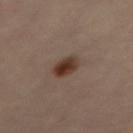Part of a total-body skin-imaging series; this lesion was reviewed on a skin check and was not flagged for biopsy.
From the abdomen.
A close-up tile cropped from a whole-body skin photograph, about 15 mm across.
A female patient in their mid- to late 30s.